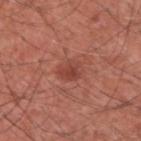{"biopsy_status": "not biopsied; imaged during a skin examination", "image": {"source": "total-body photography crop", "field_of_view_mm": 15}, "site": "upper back", "patient": {"sex": "male", "age_approx": 60}}